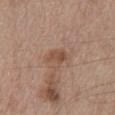The lesion was tiled from a total-body skin photograph and was not biopsied. The lesion is located on the front of the torso. Cropped from a whole-body photographic skin survey; the tile spans about 15 mm. Imaged with white-light lighting. The total-body-photography lesion software estimated an outline eccentricity of about 0.75 (0 = round, 1 = elongated). It also reported a mean CIELAB color near L≈50 a*≈19 b*≈28, a lesion–skin lightness drop of about 8, and a normalized border contrast of about 6.5. The software also gave a border-irregularity index near 2.5/10 and internal color variation of about 3 on a 0–10 scale. A male subject aged around 70. The lesion's longest dimension is about 2.5 mm.